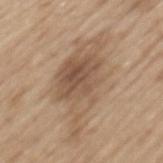No biopsy was performed on this lesion — it was imaged during a full skin examination and was not determined to be concerning.
This is a white-light tile.
Measured at roughly 10 mm in maximum diameter.
From the mid back.
A roughly 15 mm field-of-view crop from a total-body skin photograph.
A male patient aged 68–72.